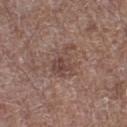No biopsy was performed on this lesion — it was imaged during a full skin examination and was not determined to be concerning.
Automated image analysis of the tile measured a color-variation rating of about 4.5/10. The software also gave a classifier nevus-likeness of about 0/100 and a detector confidence of about 100 out of 100 that the crop contains a lesion.
Approximately 4 mm at its widest.
The subject is a male aged 68–72.
On the leg.
A 15 mm close-up extracted from a 3D total-body photography capture.